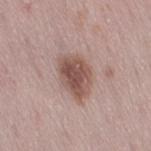workup = catalogued during a skin exam; not biopsied
body site = the right thigh
patient = female, aged 28–32
image source = ~15 mm tile from a whole-body skin photo